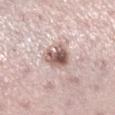This lesion was catalogued during total-body skin photography and was not selected for biopsy.
This is a white-light tile.
The lesion's longest dimension is about 3.5 mm.
This image is a 15 mm lesion crop taken from a total-body photograph.
The lesion is located on the right lower leg.
The patient is a female aged 33–37.